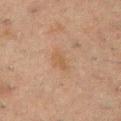The lesion was tiled from a total-body skin photograph and was not biopsied. The lesion's longest dimension is about 3 mm. Located on the chest. The patient is a male approximately 50 years of age. Cropped from a total-body skin-imaging series; the visible field is about 15 mm.